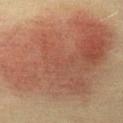Impression: Captured during whole-body skin photography for melanoma surveillance; the lesion was not biopsied. Context: This is a cross-polarized tile. A region of skin cropped from a whole-body photographic capture, roughly 15 mm wide. Located on the abdomen. The lesion's longest dimension is about 15.5 mm. A female subject, in their mid-50s.